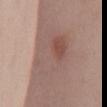workup: catalogued during a skin exam; not biopsied
subject: female, aged approximately 40
image source: total-body-photography crop, ~15 mm field of view
automated lesion analysis: a lesion area of about 25 mm², an eccentricity of roughly 0.8, and a shape-asymmetry score of about 0.25 (0 = symmetric); a classifier nevus-likeness of about 0/100 and lesion-presence confidence of about 95/100
location: the mid back
lighting: white-light illumination